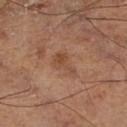The lesion-visualizer software estimated a lesion area of about 5.5 mm², an outline eccentricity of about 0.85 (0 = round, 1 = elongated), and a symmetry-axis asymmetry near 0.5. The analysis additionally found border irregularity of about 5.5 on a 0–10 scale, a color-variation rating of about 2/10, and peripheral color asymmetry of about 1. It also reported a classifier nevus-likeness of about 0/100 and a lesion-detection confidence of about 100/100.
The lesion is located on the right thigh.
This image is a 15 mm lesion crop taken from a total-body photograph.